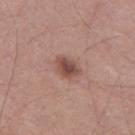Assessment:
The lesion was photographed on a routine skin check and not biopsied; there is no pathology result.
Clinical summary:
The subject is a male aged 58–62. On the left thigh. A 15 mm close-up extracted from a 3D total-body photography capture.